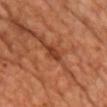The lesion was photographed on a routine skin check and not biopsied; there is no pathology result. From the chest. The subject is a male aged around 70. Cropped from a whole-body photographic skin survey; the tile spans about 15 mm. This is a cross-polarized tile. Automated image analysis of the tile measured an area of roughly 2.5 mm², an eccentricity of roughly 0.9, and a shape-asymmetry score of about 0.3 (0 = symmetric). Approximately 2.5 mm at its widest.